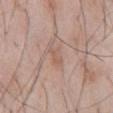• notes: catalogued during a skin exam; not biopsied
• patient: male, approximately 55 years of age
• anatomic site: the abdomen
• image source: ~15 mm tile from a whole-body skin photo